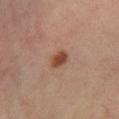This lesion was catalogued during total-body skin photography and was not selected for biopsy.
On the right lower leg.
A male patient aged 53–57.
Measured at roughly 2.5 mm in maximum diameter.
An algorithmic analysis of the crop reported a within-lesion color-variation index near 3/10 and a peripheral color-asymmetry measure near 1. And it measured a lesion-detection confidence of about 100/100.
Cropped from a whole-body photographic skin survey; the tile spans about 15 mm.